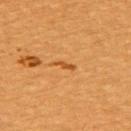Clinical impression:
Recorded during total-body skin imaging; not selected for excision or biopsy.
Acquisition and patient details:
A 15 mm close-up extracted from a 3D total-body photography capture. The recorded lesion diameter is about 2.5 mm. A female patient aged around 55. The lesion is on the upper back.